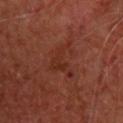The lesion was tiled from a total-body skin photograph and was not biopsied.
Imaged with cross-polarized lighting.
Cropped from a whole-body photographic skin survey; the tile spans about 15 mm.
The lesion-visualizer software estimated a footprint of about 8 mm², an eccentricity of roughly 0.55, and two-axis asymmetry of about 0.6. The software also gave an average lesion color of about L≈26 a*≈23 b*≈25 (CIELAB) and a lesion–skin lightness drop of about 5. And it measured border irregularity of about 8 on a 0–10 scale and radial color variation of about 0.5. It also reported lesion-presence confidence of about 95/100.
A male subject in their mid-60s.
Located on the upper back.
The recorded lesion diameter is about 4.5 mm.